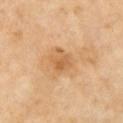{"biopsy_status": "not biopsied; imaged during a skin examination", "image": {"source": "total-body photography crop", "field_of_view_mm": 15}, "lighting": "cross-polarized", "site": "right upper arm", "lesion_size": {"long_diameter_mm_approx": 3.5}, "patient": {"sex": "female", "age_approx": 70}}